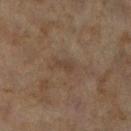Recorded during total-body skin imaging; not selected for excision or biopsy. A female patient aged 58 to 62. The lesion-visualizer software estimated a lesion area of about 3 mm², an eccentricity of roughly 0.85, and a shape-asymmetry score of about 0.3 (0 = symmetric). The analysis additionally found a border-irregularity index near 3/10 and peripheral color asymmetry of about 0.5. On the right lower leg. Measured at roughly 2.5 mm in maximum diameter. A 15 mm close-up extracted from a 3D total-body photography capture. Imaged with cross-polarized lighting.